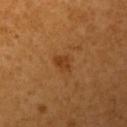notes = imaged on a skin check; not biopsied | image source = ~15 mm crop, total-body skin-cancer survey | location = the left upper arm | tile lighting = cross-polarized | lesion diameter = ~2.5 mm (longest diameter) | subject = female, about 55 years old.